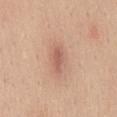The lesion was tiled from a total-body skin photograph and was not biopsied. A lesion tile, about 15 mm wide, cut from a 3D total-body photograph. From the mid back. Captured under white-light illumination. The lesion-visualizer software estimated an area of roughly 3.5 mm², a shape eccentricity near 0.9, and a shape-asymmetry score of about 0.15 (0 = symmetric). The software also gave a mean CIELAB color near L≈58 a*≈23 b*≈28, about 10 CIELAB-L* units darker than the surrounding skin, and a lesion-to-skin contrast of about 6.5 (normalized; higher = more distinct). It also reported a nevus-likeness score of about 35/100. The lesion's longest dimension is about 3 mm. The patient is a male approximately 30 years of age.